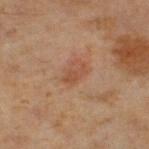Q: Was this lesion biopsied?
A: no biopsy performed (imaged during a skin exam)
Q: Automated lesion metrics?
A: a lesion area of about 3 mm² and two-axis asymmetry of about 0.35; border irregularity of about 4 on a 0–10 scale, internal color variation of about 0.5 on a 0–10 scale, and peripheral color asymmetry of about 0; a nevus-likeness score of about 45/100
Q: How was this image acquired?
A: ~15 mm crop, total-body skin-cancer survey
Q: Lesion size?
A: ≈2.5 mm
Q: What lighting was used for the tile?
A: cross-polarized illumination
Q: Who is the patient?
A: male, about 60 years old
Q: Lesion location?
A: the right lower leg